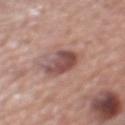Automated tile analysis of the lesion measured a footprint of about 9.5 mm² and an eccentricity of roughly 0.9. The software also gave a border-irregularity index near 3/10, a within-lesion color-variation index near 5/10, and radial color variation of about 2. Measured at roughly 5.5 mm in maximum diameter. On the mid back. This is a white-light tile. A 15 mm close-up extracted from a 3D total-body photography capture. A male patient roughly 75 years of age.